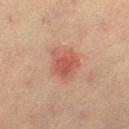Captured under cross-polarized illumination.
A roughly 15 mm field-of-view crop from a total-body skin photograph.
The recorded lesion diameter is about 4.5 mm.
Automated image analysis of the tile measured a mean CIELAB color near L≈49 a*≈24 b*≈27 and a lesion-to-skin contrast of about 6.5 (normalized; higher = more distinct).
A male patient in their mid-50s.
On the left thigh.